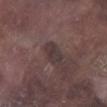site — the leg; imaging modality — ~15 mm tile from a whole-body skin photo; illumination — white-light; lesion size — ≈3 mm; subject — male, in their mid-70s.